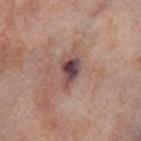The lesion was tiled from a total-body skin photograph and was not biopsied. From the right thigh. A female subject approximately 55 years of age. Cropped from a total-body skin-imaging series; the visible field is about 15 mm. Approximately 3.5 mm at its widest. The tile uses cross-polarized illumination.